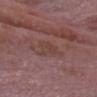Impression: Part of a total-body skin-imaging series; this lesion was reviewed on a skin check and was not flagged for biopsy. Image and clinical context: A region of skin cropped from a whole-body photographic capture, roughly 15 mm wide. On the head or neck. A male subject, in their mid-60s.